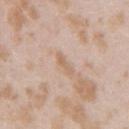Notes:
• workup: catalogued during a skin exam; not biopsied
• image source: ~15 mm crop, total-body skin-cancer survey
• patient: female, aged around 25
• body site: the right upper arm
• lesion diameter: ≈3 mm
• image-analysis metrics: roughly 7 lightness units darker than nearby skin and a normalized lesion–skin contrast near 6; an automated nevus-likeness rating near 0 out of 100 and a detector confidence of about 95 out of 100 that the crop contains a lesion
• illumination: white-light